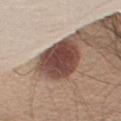A male patient, about 60 years old. Automated image analysis of the tile measured an area of roughly 19 mm² and two-axis asymmetry of about 0.2. The analysis additionally found about 20 CIELAB-L* units darker than the surrounding skin and a normalized border contrast of about 14. The analysis additionally found a border-irregularity rating of about 2/10. It also reported a lesion-detection confidence of about 100/100. On the right upper arm. The lesion's longest dimension is about 5.5 mm. A region of skin cropped from a whole-body photographic capture, roughly 15 mm wide. The tile uses white-light illumination.